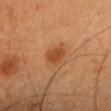Case summary:
* notes — total-body-photography surveillance lesion; no biopsy
* automated metrics — a lesion color around L≈41 a*≈23 b*≈34 in CIELAB, a lesion–skin lightness drop of about 8, and a normalized lesion–skin contrast near 7.5; border irregularity of about 2 on a 0–10 scale and a color-variation rating of about 1/10
* image — ~15 mm crop, total-body skin-cancer survey
* lesion size — about 3 mm
* anatomic site — the head or neck
* subject — female, aged around 40